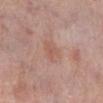| field | value |
|---|---|
| biopsy status | imaged on a skin check; not biopsied |
| image | 15 mm crop, total-body photography |
| subject | female, aged 53 to 57 |
| lighting | white-light |
| anatomic site | the right lower leg |
| diameter | ~3 mm (longest diameter) |
| automated metrics | an area of roughly 5.5 mm²; a color-variation rating of about 1.5/10 and peripheral color asymmetry of about 0.5 |